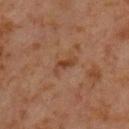Q: What did automated image analysis measure?
A: a lesion–skin lightness drop of about 7; internal color variation of about 1.5 on a 0–10 scale and a peripheral color-asymmetry measure near 0; lesion-presence confidence of about 100/100
Q: What are the patient's age and sex?
A: male, approximately 60 years of age
Q: What kind of image is this?
A: 15 mm crop, total-body photography
Q: Lesion size?
A: about 3 mm
Q: What is the anatomic site?
A: the chest
Q: What lighting was used for the tile?
A: cross-polarized